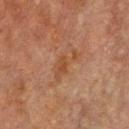Impression:
The lesion was photographed on a routine skin check and not biopsied; there is no pathology result.
Background:
Cropped from a total-body skin-imaging series; the visible field is about 15 mm. The lesion is on the front of the torso. A male patient, aged 68 to 72.